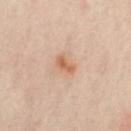Clinical impression:
Recorded during total-body skin imaging; not selected for excision or biopsy.
Background:
The tile uses cross-polarized illumination. From the abdomen. Cropped from a total-body skin-imaging series; the visible field is about 15 mm. The subject is a female aged approximately 40.